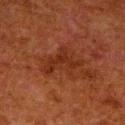This lesion was catalogued during total-body skin photography and was not selected for biopsy.
A male patient, roughly 80 years of age.
Automated image analysis of the tile measured a lesion area of about 9.5 mm², an eccentricity of roughly 0.8, and a shape-asymmetry score of about 0.55 (0 = symmetric).
Located on the right lower leg.
Longest diameter approximately 5 mm.
The tile uses cross-polarized illumination.
This image is a 15 mm lesion crop taken from a total-body photograph.